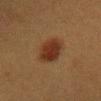Impression: No biopsy was performed on this lesion — it was imaged during a full skin examination and was not determined to be concerning. Background: Measured at roughly 4 mm in maximum diameter. The patient is a female in their 40s. A region of skin cropped from a whole-body photographic capture, roughly 15 mm wide. The tile uses cross-polarized illumination. On the abdomen. An algorithmic analysis of the crop reported a footprint of about 10 mm² and an outline eccentricity of about 0.6 (0 = round, 1 = elongated). The analysis additionally found about 9 CIELAB-L* units darker than the surrounding skin and a normalized border contrast of about 9.5. The software also gave border irregularity of about 1.5 on a 0–10 scale, internal color variation of about 3 on a 0–10 scale, and radial color variation of about 1. The software also gave a classifier nevus-likeness of about 100/100.